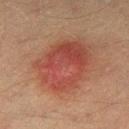The lesion was tiled from a total-body skin photograph and was not biopsied.
A 15 mm close-up tile from a total-body photography series done for melanoma screening.
The subject is a male about 40 years old.
From the right thigh.